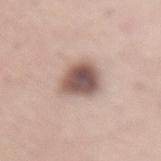Impression: The lesion was photographed on a routine skin check and not biopsied; there is no pathology result. Background: Longest diameter approximately 4 mm. Imaged with white-light lighting. A 15 mm close-up tile from a total-body photography series done for melanoma screening. On the lower back. The subject is a male roughly 55 years of age.